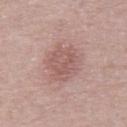No biopsy was performed on this lesion — it was imaged during a full skin examination and was not determined to be concerning. The lesion is on the chest. This image is a 15 mm lesion crop taken from a total-body photograph. A male subject, aged 63 to 67. The lesion's longest dimension is about 6 mm. The tile uses white-light illumination. Automated tile analysis of the lesion measured an area of roughly 17 mm² and a shape-asymmetry score of about 0.15 (0 = symmetric). It also reported a lesion color around L≈57 a*≈20 b*≈22 in CIELAB, about 9 CIELAB-L* units darker than the surrounding skin, and a normalized border contrast of about 6.